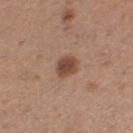The lesion was tiled from a total-body skin photograph and was not biopsied. An algorithmic analysis of the crop reported a symmetry-axis asymmetry near 0.2. And it measured a mean CIELAB color near L≈46 a*≈20 b*≈28 and about 12 CIELAB-L* units darker than the surrounding skin. Cropped from a whole-body photographic skin survey; the tile spans about 15 mm. Approximately 2.5 mm at its widest. The lesion is located on the left thigh. A female patient, aged 28–32.